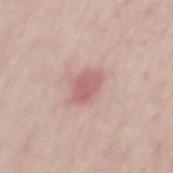No biopsy was performed on this lesion — it was imaged during a full skin examination and was not determined to be concerning.
The lesion's longest dimension is about 3.5 mm.
A male subject, aged 48 to 52.
A close-up tile cropped from a whole-body skin photograph, about 15 mm across.
On the mid back.
The lesion-visualizer software estimated an area of roughly 5.5 mm², an eccentricity of roughly 0.8, and a shape-asymmetry score of about 0.2 (0 = symmetric). The analysis additionally found border irregularity of about 2 on a 0–10 scale, a within-lesion color-variation index near 2.5/10, and a peripheral color-asymmetry measure near 1. And it measured a nevus-likeness score of about 0/100 and lesion-presence confidence of about 100/100.
This is a white-light tile.